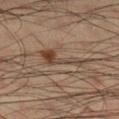{
  "lesion_size": {
    "long_diameter_mm_approx": 5.5
  },
  "patient": {
    "sex": "male",
    "age_approx": 35
  },
  "site": "right lower leg",
  "image": {
    "source": "total-body photography crop",
    "field_of_view_mm": 15
  },
  "lighting": "cross-polarized",
  "automated_metrics": {
    "area_mm2_approx": 7.5,
    "eccentricity": 0.95,
    "shape_asymmetry": 0.65,
    "border_irregularity_0_10": 8.5,
    "peripheral_color_asymmetry": 1.0
  }
}